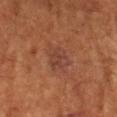Part of a total-body skin-imaging series; this lesion was reviewed on a skin check and was not flagged for biopsy. A region of skin cropped from a whole-body photographic capture, roughly 15 mm wide. The tile uses cross-polarized illumination. From the arm. The subject is a female approximately 80 years of age. The recorded lesion diameter is about 3.5 mm.